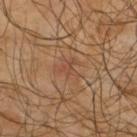Captured during whole-body skin photography for melanoma surveillance; the lesion was not biopsied. Cropped from a whole-body photographic skin survey; the tile spans about 15 mm. Captured under cross-polarized illumination. Longest diameter approximately 3 mm. A male subject aged around 65. Located on the upper back. Automated image analysis of the tile measured an area of roughly 4 mm², an eccentricity of roughly 0.45, and two-axis asymmetry of about 0.55. The analysis additionally found border irregularity of about 6 on a 0–10 scale and a peripheral color-asymmetry measure near 0.5.